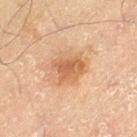Recorded during total-body skin imaging; not selected for excision or biopsy. A 15 mm crop from a total-body photograph taken for skin-cancer surveillance. Captured under cross-polarized illumination. The subject is a male roughly 70 years of age. The lesion is located on the left thigh. The recorded lesion diameter is about 4 mm. An algorithmic analysis of the crop reported a footprint of about 8 mm², an outline eccentricity of about 0.7 (0 = round, 1 = elongated), and a shape-asymmetry score of about 0.35 (0 = symmetric). The software also gave a border-irregularity rating of about 3.5/10 and a peripheral color-asymmetry measure near 1. It also reported a nevus-likeness score of about 55/100 and a lesion-detection confidence of about 100/100.